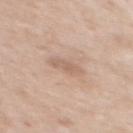Notes:
* subject — female, in their 50s
* tile lighting — white-light
* body site — the upper back
* image source — 15 mm crop, total-body photography
* lesion diameter — ≈3 mm